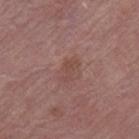Imaged during a routine full-body skin examination; the lesion was not biopsied and no histopathology is available. A roughly 15 mm field-of-view crop from a total-body skin photograph. A female patient, approximately 70 years of age. From the right thigh. The total-body-photography lesion software estimated about 6 CIELAB-L* units darker than the surrounding skin and a normalized border contrast of about 5. The analysis additionally found internal color variation of about 1 on a 0–10 scale. The software also gave a nevus-likeness score of about 0/100 and lesion-presence confidence of about 100/100. The lesion's longest dimension is about 3 mm. The tile uses white-light illumination.